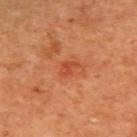No biopsy was performed on this lesion — it was imaged during a full skin examination and was not determined to be concerning. The lesion is located on the upper back. Longest diameter approximately 2.5 mm. An algorithmic analysis of the crop reported an average lesion color of about L≈50 a*≈34 b*≈40 (CIELAB), roughly 8 lightness units darker than nearby skin, and a normalized border contrast of about 6. And it measured a border-irregularity rating of about 4/10, internal color variation of about 1.5 on a 0–10 scale, and radial color variation of about 0.5. The software also gave an automated nevus-likeness rating near 50 out of 100 and lesion-presence confidence of about 100/100. A female subject aged 38–42. A 15 mm close-up extracted from a 3D total-body photography capture. Captured under cross-polarized illumination.